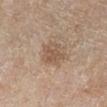Q: Was a biopsy performed?
A: total-body-photography surveillance lesion; no biopsy
Q: What is the imaging modality?
A: ~15 mm tile from a whole-body skin photo
Q: Patient demographics?
A: female, aged 58–62
Q: Illumination type?
A: cross-polarized illumination
Q: What is the lesion's diameter?
A: ≈3 mm
Q: Lesion location?
A: the right lower leg
Q: What did automated image analysis measure?
A: a within-lesion color-variation index near 2.5/10